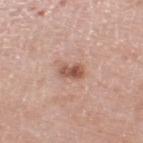Acquisition and patient details:
A roughly 15 mm field-of-view crop from a total-body skin photograph. The patient is a male aged 78 to 82. On the right thigh.